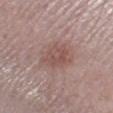This lesion was catalogued during total-body skin photography and was not selected for biopsy. Automated tile analysis of the lesion measured a border-irregularity rating of about 3.5/10, a within-lesion color-variation index near 3/10, and peripheral color asymmetry of about 1. And it measured an automated nevus-likeness rating near 30 out of 100 and a lesion-detection confidence of about 100/100. This image is a 15 mm lesion crop taken from a total-body photograph. On the left leg. The recorded lesion diameter is about 4 mm. A female patient, in their mid- to late 60s.